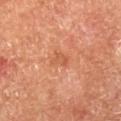Part of a total-body skin-imaging series; this lesion was reviewed on a skin check and was not flagged for biopsy.
A 15 mm close-up extracted from a 3D total-body photography capture.
The recorded lesion diameter is about 2.5 mm.
A male subject aged around 65.
The tile uses cross-polarized illumination.
Automated image analysis of the tile measured a mean CIELAB color near L≈57 a*≈28 b*≈38, about 7 CIELAB-L* units darker than the surrounding skin, and a normalized lesion–skin contrast near 5. The analysis additionally found a classifier nevus-likeness of about 0/100 and a detector confidence of about 100 out of 100 that the crop contains a lesion.
The lesion is located on the right lower leg.